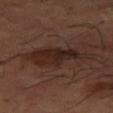Impression: No biopsy was performed on this lesion — it was imaged during a full skin examination and was not determined to be concerning. Acquisition and patient details: The subject is a male aged 63 to 67. Cropped from a total-body skin-imaging series; the visible field is about 15 mm. Imaged with cross-polarized lighting. The lesion's longest dimension is about 7.5 mm. Located on the right thigh.